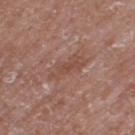Imaged during a routine full-body skin examination; the lesion was not biopsied and no histopathology is available.
A roughly 15 mm field-of-view crop from a total-body skin photograph.
The subject is a male aged 58 to 62.
The lesion's longest dimension is about 5 mm.
From the right thigh.